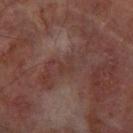follow-up: total-body-photography surveillance lesion; no biopsy
illumination: cross-polarized illumination
lesion diameter: about 3 mm
anatomic site: the left forearm
subject: male, approximately 65 years of age
TBP lesion metrics: an area of roughly 3 mm², an outline eccentricity of about 0.8 (0 = round, 1 = elongated), and two-axis asymmetry of about 0.4; border irregularity of about 4.5 on a 0–10 scale, a within-lesion color-variation index near 0/10, and a peripheral color-asymmetry measure near 0
image source: 15 mm crop, total-body photography